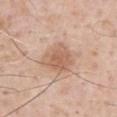<case>
<biopsy_status>not biopsied; imaged during a skin examination</biopsy_status>
<image>
  <source>total-body photography crop</source>
  <field_of_view_mm>15</field_of_view_mm>
</image>
<patient>
  <sex>male</sex>
  <age_approx>75</age_approx>
</patient>
<site>abdomen</site>
</case>